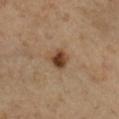notes: imaged on a skin check; not biopsied | lighting: cross-polarized | site: the right lower leg | subject: male, in their mid- to late 60s | imaging modality: total-body-photography crop, ~15 mm field of view | TBP lesion metrics: an outline eccentricity of about 0.25 (0 = round, 1 = elongated) and two-axis asymmetry of about 0.15; an average lesion color of about L≈42 a*≈19 b*≈31 (CIELAB) and a lesion-to-skin contrast of about 11 (normalized; higher = more distinct); an automated nevus-likeness rating near 100 out of 100 and a lesion-detection confidence of about 100/100.